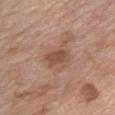lesion size — ≈3.5 mm
subject — female, in their mid- to late 70s
image-analysis metrics — a lesion area of about 6 mm², an eccentricity of roughly 0.8, and two-axis asymmetry of about 0.25; an average lesion color of about L≈50 a*≈20 b*≈28 (CIELAB), a lesion–skin lightness drop of about 9, and a normalized lesion–skin contrast near 6.5
body site — the chest
lighting — white-light
image — ~15 mm tile from a whole-body skin photo